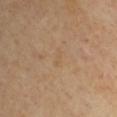Part of a total-body skin-imaging series; this lesion was reviewed on a skin check and was not flagged for biopsy. A roughly 15 mm field-of-view crop from a total-body skin photograph. On the left upper arm. A male subject, about 55 years old.